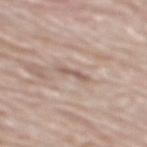Q: Was a biopsy performed?
A: catalogued during a skin exam; not biopsied
Q: Automated lesion metrics?
A: border irregularity of about 4.5 on a 0–10 scale and radial color variation of about 0; a nevus-likeness score of about 0/100 and a detector confidence of about 70 out of 100 that the crop contains a lesion
Q: Lesion size?
A: about 2.5 mm
Q: Where on the body is the lesion?
A: the mid back
Q: What kind of image is this?
A: total-body-photography crop, ~15 mm field of view
Q: Patient demographics?
A: male, approximately 80 years of age